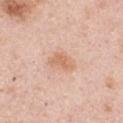Imaged during a routine full-body skin examination; the lesion was not biopsied and no histopathology is available.
Cropped from a total-body skin-imaging series; the visible field is about 15 mm.
On the chest.
The lesion-visualizer software estimated a border-irregularity rating of about 2.5/10, internal color variation of about 3 on a 0–10 scale, and peripheral color asymmetry of about 1.
The tile uses white-light illumination.
A male patient approximately 50 years of age.